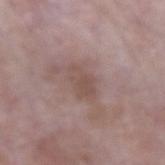{
  "biopsy_status": "not biopsied; imaged during a skin examination",
  "site": "left forearm",
  "patient": {
    "sex": "male",
    "age_approx": 65
  },
  "image": {
    "source": "total-body photography crop",
    "field_of_view_mm": 15
  }
}